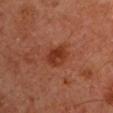workup: imaged on a skin check; not biopsied | acquisition: ~15 mm tile from a whole-body skin photo | subject: male, approximately 65 years of age | automated lesion analysis: a shape eccentricity near 0.75 and a shape-asymmetry score of about 0.15 (0 = symmetric); an average lesion color of about L≈31 a*≈25 b*≈29 (CIELAB), roughly 8 lightness units darker than nearby skin, and a normalized lesion–skin contrast near 8 | lighting: cross-polarized illumination | body site: the right upper arm.